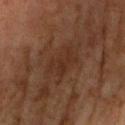| feature | finding |
|---|---|
| anatomic site | the right upper arm |
| acquisition | ~15 mm tile from a whole-body skin photo |
| patient | female, aged approximately 60 |